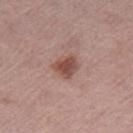Q: Is there a histopathology result?
A: no biopsy performed (imaged during a skin exam)
Q: Lesion location?
A: the right thigh
Q: How was this image acquired?
A: ~15 mm tile from a whole-body skin photo
Q: What are the patient's age and sex?
A: female, in their mid- to late 60s